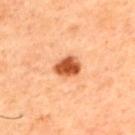Image and clinical context: The recorded lesion diameter is about 3 mm. Automated image analysis of the tile measured an area of roughly 6 mm², an outline eccentricity of about 0.65 (0 = round, 1 = elongated), and a shape-asymmetry score of about 0.2 (0 = symmetric). And it measured a classifier nevus-likeness of about 100/100 and lesion-presence confidence of about 100/100. A 15 mm close-up tile from a total-body photography series done for melanoma screening. On the back. A male subject aged around 45.